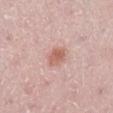Assessment:
Recorded during total-body skin imaging; not selected for excision or biopsy.
Clinical summary:
A female patient in their mid- to late 20s. The lesion is located on the leg. Captured under white-light illumination. A roughly 15 mm field-of-view crop from a total-body skin photograph.